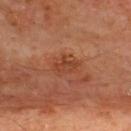workup: total-body-photography surveillance lesion; no biopsy
patient: male, aged 63 to 67
lighting: cross-polarized illumination
diameter: ≈3.5 mm
image source: total-body-photography crop, ~15 mm field of view
body site: the upper back